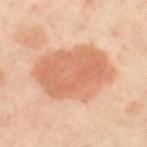Assessment:
No biopsy was performed on this lesion — it was imaged during a full skin examination and was not determined to be concerning.
Image and clinical context:
Captured under cross-polarized illumination. A female subject aged around 50. A close-up tile cropped from a whole-body skin photograph, about 15 mm across. Longest diameter approximately 8.5 mm. An algorithmic analysis of the crop reported a mean CIELAB color near L≈67 a*≈26 b*≈36, about 11 CIELAB-L* units darker than the surrounding skin, and a lesion-to-skin contrast of about 7 (normalized; higher = more distinct). The analysis additionally found a classifier nevus-likeness of about 95/100 and a detector confidence of about 100 out of 100 that the crop contains a lesion. Located on the right thigh.